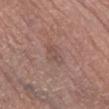Captured during whole-body skin photography for melanoma surveillance; the lesion was not biopsied.
A 15 mm close-up extracted from a 3D total-body photography capture.
Automated tile analysis of the lesion measured an eccentricity of roughly 0.7 and a shape-asymmetry score of about 0.25 (0 = symmetric). The software also gave a mean CIELAB color near L≈50 a*≈18 b*≈22, about 6 CIELAB-L* units darker than the surrounding skin, and a normalized lesion–skin contrast near 4.5. The analysis additionally found a border-irregularity rating of about 3.5/10 and radial color variation of about 1.5.
The subject is a male roughly 70 years of age.
The lesion is located on the leg.